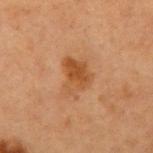Q: Is there a histopathology result?
A: total-body-photography surveillance lesion; no biopsy
Q: Illumination type?
A: cross-polarized illumination
Q: What is the lesion's diameter?
A: ~4.5 mm (longest diameter)
Q: Who is the patient?
A: female, approximately 40 years of age
Q: What kind of image is this?
A: total-body-photography crop, ~15 mm field of view
Q: Lesion location?
A: the right upper arm